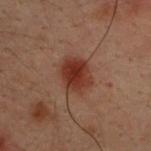The lesion was tiled from a total-body skin photograph and was not biopsied. A roughly 15 mm field-of-view crop from a total-body skin photograph. The lesion-visualizer software estimated a footprint of about 11 mm² and a shape eccentricity near 0.65. The software also gave about 9 CIELAB-L* units darker than the surrounding skin and a normalized border contrast of about 9.5. The software also gave border irregularity of about 1.5 on a 0–10 scale, a within-lesion color-variation index near 3.5/10, and radial color variation of about 1. A male subject aged around 30. The lesion is on the back. Captured under cross-polarized illumination.